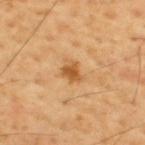Part of a total-body skin-imaging series; this lesion was reviewed on a skin check and was not flagged for biopsy.
A male subject, aged 53–57.
A 15 mm crop from a total-body photograph taken for skin-cancer surveillance.
The lesion is located on the upper back.